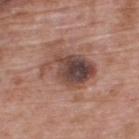{"patient": {"sex": "male", "age_approx": 70}, "automated_metrics": {"border_irregularity_0_10": 4.5, "color_variation_0_10": 10.0, "nevus_likeness_0_100": 10, "lesion_detection_confidence_0_100": 100}, "site": "upper back", "lesion_size": {"long_diameter_mm_approx": 6.5}, "lighting": "white-light", "image": {"source": "total-body photography crop", "field_of_view_mm": 15}}